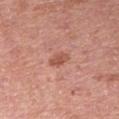Captured during whole-body skin photography for melanoma surveillance; the lesion was not biopsied.
On the arm.
Cropped from a whole-body photographic skin survey; the tile spans about 15 mm.
The subject is a female approximately 40 years of age.